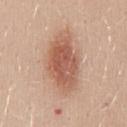Context:
Cropped from a total-body skin-imaging series; the visible field is about 15 mm. A female subject aged 28 to 32. This is a white-light tile. Automated image analysis of the tile measured a lesion area of about 24 mm², a shape eccentricity near 0.85, and a symmetry-axis asymmetry near 0.2. And it measured a mean CIELAB color near L≈58 a*≈22 b*≈30 and roughly 12 lightness units darker than nearby skin. The software also gave border irregularity of about 3 on a 0–10 scale. About 8 mm across. On the back.